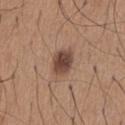Captured during whole-body skin photography for melanoma surveillance; the lesion was not biopsied.
This is a white-light tile.
The subject is a male roughly 65 years of age.
A 15 mm close-up tile from a total-body photography series done for melanoma screening.
Automated tile analysis of the lesion measured a nevus-likeness score of about 85/100 and lesion-presence confidence of about 100/100.
Located on the chest.